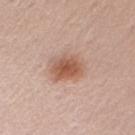Impression: Recorded during total-body skin imaging; not selected for excision or biopsy. Context: The lesion is located on the arm. Cropped from a total-body skin-imaging series; the visible field is about 15 mm. Captured under white-light illumination. The lesion's longest dimension is about 3.5 mm. A female subject, roughly 30 years of age.